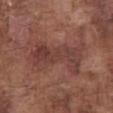Background:
This is a white-light tile. The lesion is located on the front of the torso. Cropped from a total-body skin-imaging series; the visible field is about 15 mm. A male patient in their mid-70s. The recorded lesion diameter is about 8 mm.